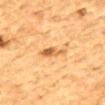follow-up: catalogued during a skin exam; not biopsied
patient: male, aged 83 or over
image-analysis metrics: a lesion area of about 4.5 mm², an outline eccentricity of about 0.85 (0 = round, 1 = elongated), and a shape-asymmetry score of about 0.3 (0 = symmetric); an average lesion color of about L≈56 a*≈22 b*≈41 (CIELAB); an automated nevus-likeness rating near 60 out of 100 and lesion-presence confidence of about 100/100
diameter: about 3.5 mm
lighting: cross-polarized illumination
acquisition: total-body-photography crop, ~15 mm field of view
site: the mid back